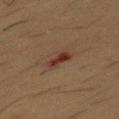Impression: No biopsy was performed on this lesion — it was imaged during a full skin examination and was not determined to be concerning. Background: A roughly 15 mm field-of-view crop from a total-body skin photograph. Measured at roughly 2.5 mm in maximum diameter. The total-body-photography lesion software estimated a lesion area of about 4 mm², an outline eccentricity of about 0.8 (0 = round, 1 = elongated), and two-axis asymmetry of about 0.3. On the chest. The patient is a male approximately 55 years of age.